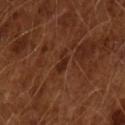Q: Was a biopsy performed?
A: total-body-photography surveillance lesion; no biopsy